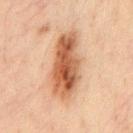Findings:
– workup — imaged on a skin check; not biopsied
– lesion diameter — ~8 mm (longest diameter)
– TBP lesion metrics — an average lesion color of about L≈53 a*≈23 b*≈34 (CIELAB) and a lesion-to-skin contrast of about 10.5 (normalized; higher = more distinct); internal color variation of about 8 on a 0–10 scale and a peripheral color-asymmetry measure near 3; an automated nevus-likeness rating near 95 out of 100 and lesion-presence confidence of about 100/100
– illumination — cross-polarized
– patient — male, approximately 35 years of age
– site — the chest
– acquisition — ~15 mm tile from a whole-body skin photo